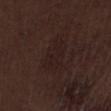Impression:
Imaged during a routine full-body skin examination; the lesion was not biopsied and no histopathology is available.
Background:
This image is a 15 mm lesion crop taken from a total-body photograph. From the leg. Imaged with white-light lighting. Longest diameter approximately 5 mm. Automated image analysis of the tile measured a lesion area of about 9.5 mm², an outline eccentricity of about 0.85 (0 = round, 1 = elongated), and a shape-asymmetry score of about 0.35 (0 = symmetric). It also reported roughly 3 lightness units darker than nearby skin and a normalized lesion–skin contrast near 4.5. And it measured a border-irregularity rating of about 4.5/10. A male patient, aged 68 to 72.